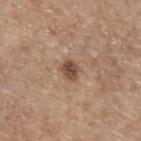This lesion was catalogued during total-body skin photography and was not selected for biopsy. The lesion is located on the left forearm. The lesion-visualizer software estimated an average lesion color of about L≈48 a*≈18 b*≈28 (CIELAB), roughly 12 lightness units darker than nearby skin, and a normalized lesion–skin contrast near 9. The software also gave a peripheral color-asymmetry measure near 1. The patient is a female in their mid- to late 60s. The recorded lesion diameter is about 2.5 mm. A region of skin cropped from a whole-body photographic capture, roughly 15 mm wide.